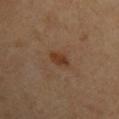illumination: cross-polarized
diameter: ≈2.5 mm
TBP lesion metrics: a lesion–skin lightness drop of about 8 and a lesion-to-skin contrast of about 8 (normalized; higher = more distinct); a classifier nevus-likeness of about 85/100 and a lesion-detection confidence of about 100/100
image: ~15 mm tile from a whole-body skin photo
site: the arm
subject: male, about 60 years old